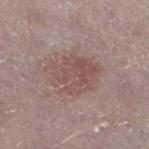The lesion was tiled from a total-body skin photograph and was not biopsied. The patient is a male aged approximately 75. The recorded lesion diameter is about 5.5 mm. A 15 mm close-up tile from a total-body photography series done for melanoma screening. The lesion-visualizer software estimated a lesion area of about 17 mm², an eccentricity of roughly 0.7, and a shape-asymmetry score of about 0.2 (0 = symmetric). The analysis additionally found a nevus-likeness score of about 10/100 and lesion-presence confidence of about 100/100. Located on the right thigh.